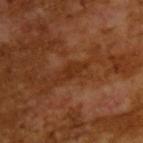This lesion was catalogued during total-body skin photography and was not selected for biopsy. Measured at roughly 3.5 mm in maximum diameter. The subject is a male aged 63–67. The lesion-visualizer software estimated a footprint of about 4.5 mm² and an eccentricity of roughly 0.9. It also reported a nevus-likeness score of about 0/100 and lesion-presence confidence of about 75/100. This is a cross-polarized tile. A 15 mm close-up extracted from a 3D total-body photography capture.